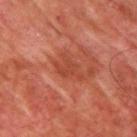Part of a total-body skin-imaging series; this lesion was reviewed on a skin check and was not flagged for biopsy. A male subject aged 63 to 67. A lesion tile, about 15 mm wide, cut from a 3D total-body photograph. This is a cross-polarized tile. On the upper back. Measured at roughly 3.5 mm in maximum diameter.